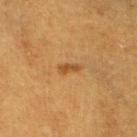Imaged during a routine full-body skin examination; the lesion was not biopsied and no histopathology is available.
Located on the left lower leg.
A 15 mm close-up tile from a total-body photography series done for melanoma screening.
A female subject in their 40s.